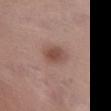The lesion was photographed on a routine skin check and not biopsied; there is no pathology result.